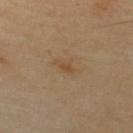No biopsy was performed on this lesion — it was imaged during a full skin examination and was not determined to be concerning.
A close-up tile cropped from a whole-body skin photograph, about 15 mm across.
A male subject about 55 years old.
On the back.
The lesion-visualizer software estimated a shape eccentricity near 0.85. The software also gave a mean CIELAB color near L≈49 a*≈16 b*≈34 and a normalized lesion–skin contrast near 6. And it measured a border-irregularity rating of about 3/10, a color-variation rating of about 1/10, and a peripheral color-asymmetry measure near 0.5.
This is a cross-polarized tile.